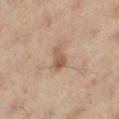* workup: total-body-photography surveillance lesion; no biopsy
* subject: female, in their mid-50s
* image source: ~15 mm tile from a whole-body skin photo
* automated lesion analysis: a classifier nevus-likeness of about 15/100 and a lesion-detection confidence of about 100/100
* illumination: cross-polarized
* site: the left leg